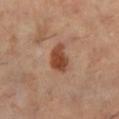Q: Was this lesion biopsied?
A: imaged on a skin check; not biopsied